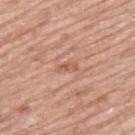The lesion was photographed on a routine skin check and not biopsied; there is no pathology result.
The patient is a male in their 80s.
The tile uses white-light illumination.
The total-body-photography lesion software estimated an automated nevus-likeness rating near 0 out of 100.
Cropped from a total-body skin-imaging series; the visible field is about 15 mm.
From the upper back.
Approximately 2.5 mm at its widest.